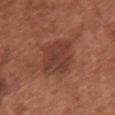biopsy status = total-body-photography surveillance lesion; no biopsy | lighting = white-light | acquisition = ~15 mm tile from a whole-body skin photo | location = the front of the torso | subject = female, in their mid-60s | lesion diameter = about 4.5 mm.